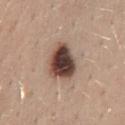The lesion was tiled from a total-body skin photograph and was not biopsied. A close-up tile cropped from a whole-body skin photograph, about 15 mm across. From the abdomen. The subject is a female aged approximately 35. Automated tile analysis of the lesion measured a footprint of about 12 mm², an eccentricity of roughly 0.65, and a symmetry-axis asymmetry near 0.25. And it measured a mean CIELAB color near L≈42 a*≈18 b*≈23, a lesion–skin lightness drop of about 21, and a lesion-to-skin contrast of about 15 (normalized; higher = more distinct). The software also gave a border-irregularity index near 2.5/10, internal color variation of about 8 on a 0–10 scale, and peripheral color asymmetry of about 2. About 4.5 mm across.